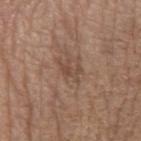Assessment: The lesion was tiled from a total-body skin photograph and was not biopsied. Clinical summary: An algorithmic analysis of the crop reported a lesion color around L≈46 a*≈18 b*≈26 in CIELAB, a lesion–skin lightness drop of about 7, and a lesion-to-skin contrast of about 5.5 (normalized; higher = more distinct). Imaged with white-light lighting. Located on the right forearm. A close-up tile cropped from a whole-body skin photograph, about 15 mm across. A female subject, in their mid- to late 70s. About 2.5 mm across.